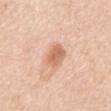<record>
  <biopsy_status>not biopsied; imaged during a skin examination</biopsy_status>
  <automated_metrics>
    <border_irregularity_0_10>2.0</border_irregularity_0_10>
    <color_variation_0_10>2.5</color_variation_0_10>
    <peripheral_color_asymmetry>1.0</peripheral_color_asymmetry>
    <lesion_detection_confidence_0_100>100</lesion_detection_confidence_0_100>
  </automated_metrics>
  <lighting>white-light</lighting>
  <site>mid back</site>
  <patient>
    <sex>female</sex>
    <age_approx>50</age_approx>
  </patient>
  <image>
    <source>total-body photography crop</source>
    <field_of_view_mm>15</field_of_view_mm>
  </image>
  <lesion_size>
    <long_diameter_mm_approx>3.5</long_diameter_mm_approx>
  </lesion_size>
</record>